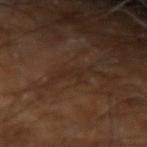Part of a total-body skin-imaging series; this lesion was reviewed on a skin check and was not flagged for biopsy. A close-up tile cropped from a whole-body skin photograph, about 15 mm across. The lesion-visualizer software estimated an average lesion color of about L≈24 a*≈15 b*≈22 (CIELAB) and roughly 4 lightness units darker than nearby skin. The software also gave a color-variation rating of about 0/10 and radial color variation of about 0. The patient is a male aged approximately 60. Approximately 4 mm at its widest. Imaged with cross-polarized lighting. From the left arm.